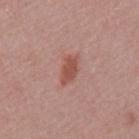A male patient in their 50s. The recorded lesion diameter is about 3.5 mm. Captured under white-light illumination. Cropped from a total-body skin-imaging series; the visible field is about 15 mm. From the mid back.